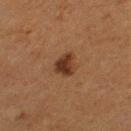Q: Was this lesion biopsied?
A: imaged on a skin check; not biopsied
Q: How was the tile lit?
A: cross-polarized illumination
Q: How was this image acquired?
A: 15 mm crop, total-body photography
Q: What did automated image analysis measure?
A: a footprint of about 5.5 mm², an eccentricity of roughly 0.6, and two-axis asymmetry of about 0.3; an automated nevus-likeness rating near 90 out of 100 and lesion-presence confidence of about 100/100
Q: Patient demographics?
A: female, aged approximately 50
Q: What is the anatomic site?
A: the left thigh
Q: Lesion size?
A: ≈3 mm